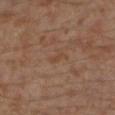Findings:
– biopsy status · imaged on a skin check; not biopsied
– lesion diameter · ≈2.5 mm
– subject · male, in their 30s
– site · the left lower leg
– image source · total-body-photography crop, ~15 mm field of view
– lighting · cross-polarized